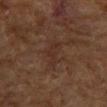biopsy status = imaged on a skin check; not biopsied | image = ~15 mm crop, total-body skin-cancer survey | anatomic site = the arm | subject = female, aged 78–82.